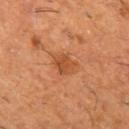Part of a total-body skin-imaging series; this lesion was reviewed on a skin check and was not flagged for biopsy.
This is a cross-polarized tile.
Cropped from a total-body skin-imaging series; the visible field is about 15 mm.
A male patient, aged around 60.
The lesion is on the left thigh.
About 3 mm across.